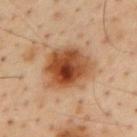Imaged during a routine full-body skin examination; the lesion was not biopsied and no histopathology is available. Imaged with cross-polarized lighting. Located on the mid back. Approximately 6 mm at its widest. A region of skin cropped from a whole-body photographic capture, roughly 15 mm wide. The patient is a male aged approximately 55.